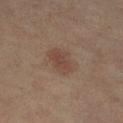Clinical summary: The lesion is located on the left lower leg. The subject is a female aged around 80. This image is a 15 mm lesion crop taken from a total-body photograph. The lesion-visualizer software estimated internal color variation of about 2.5 on a 0–10 scale and a peripheral color-asymmetry measure near 1. The recorded lesion diameter is about 3.5 mm. The tile uses cross-polarized illumination.